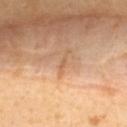Q: Was a biopsy performed?
A: total-body-photography surveillance lesion; no biopsy
Q: What lighting was used for the tile?
A: cross-polarized illumination
Q: Who is the patient?
A: female, roughly 35 years of age
Q: Where on the body is the lesion?
A: the upper back
Q: What is the lesion's diameter?
A: about 1 mm
Q: How was this image acquired?
A: ~15 mm tile from a whole-body skin photo